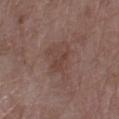image source: total-body-photography crop, ~15 mm field of view; size: ≈4.5 mm; patient: female, aged around 70; tile lighting: white-light illumination; site: the left forearm.